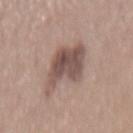{
  "biopsy_status": "not biopsied; imaged during a skin examination",
  "lighting": "white-light",
  "site": "mid back",
  "patient": {
    "sex": "female",
    "age_approx": 55
  },
  "image": {
    "source": "total-body photography crop",
    "field_of_view_mm": 15
  },
  "automated_metrics": {
    "cielab_L": 51,
    "cielab_a": 17,
    "cielab_b": 21,
    "vs_skin_darker_L": 12.0
  }
}